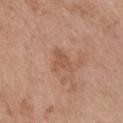The patient is a female approximately 65 years of age.
Imaged with white-light lighting.
A lesion tile, about 15 mm wide, cut from a 3D total-body photograph.
An algorithmic analysis of the crop reported a lesion color around L≈54 a*≈22 b*≈31 in CIELAB, roughly 7 lightness units darker than nearby skin, and a normalized lesion–skin contrast near 5. And it measured a nevus-likeness score of about 0/100 and a detector confidence of about 100 out of 100 that the crop contains a lesion.
Located on the chest.
Longest diameter approximately 3.5 mm.